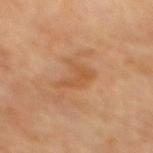  biopsy_status: not biopsied; imaged during a skin examination
  image:
    source: total-body photography crop
    field_of_view_mm: 15
  lighting: cross-polarized
  site: mid back
  lesion_size:
    long_diameter_mm_approx: 3.5
  automated_metrics:
    area_mm2_approx: 6.5
    eccentricity: 0.75
    shape_asymmetry: 0.5
    cielab_L: 53
    cielab_a: 22
    cielab_b: 38
    vs_skin_darker_L: 7.0
    vs_skin_contrast_norm: 5.5
    border_irregularity_0_10: 6.5
    color_variation_0_10: 1.5
    peripheral_color_asymmetry: 0.5
    lesion_detection_confidence_0_100: 100
  patient:
    age_approx: 65